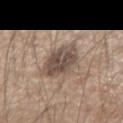| feature | finding |
|---|---|
| notes | imaged on a skin check; not biopsied |
| location | the left forearm |
| lighting | white-light |
| patient | male, aged 53–57 |
| acquisition | ~15 mm tile from a whole-body skin photo |
| image-analysis metrics | an area of roughly 14 mm², a shape eccentricity near 0.45, and two-axis asymmetry of about 0.2; a mean CIELAB color near L≈49 a*≈13 b*≈23, roughly 12 lightness units darker than nearby skin, and a lesion-to-skin contrast of about 9 (normalized; higher = more distinct); border irregularity of about 2.5 on a 0–10 scale, a color-variation rating of about 4.5/10, and peripheral color asymmetry of about 1.5 |